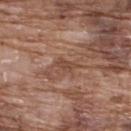Recorded during total-body skin imaging; not selected for excision or biopsy. This image is a 15 mm lesion crop taken from a total-body photograph. A male patient, aged around 75. From the back. Longest diameter approximately 2.5 mm. Imaged with white-light lighting.